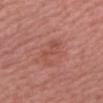Q: Is there a histopathology result?
A: imaged on a skin check; not biopsied
Q: Who is the patient?
A: female, aged approximately 70
Q: Lesion size?
A: ≈3.5 mm
Q: What is the anatomic site?
A: the left upper arm
Q: How was this image acquired?
A: 15 mm crop, total-body photography
Q: What lighting was used for the tile?
A: white-light illumination